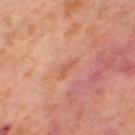Captured during whole-body skin photography for melanoma surveillance; the lesion was not biopsied. From the right thigh. A roughly 15 mm field-of-view crop from a total-body skin photograph. The patient is a female aged around 55. This is a cross-polarized tile. About 3 mm across.